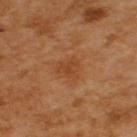This lesion was catalogued during total-body skin photography and was not selected for biopsy. The lesion is on the arm. Cropped from a whole-body photographic skin survey; the tile spans about 15 mm. A female patient aged around 55. The recorded lesion diameter is about 3 mm. The tile uses cross-polarized illumination.